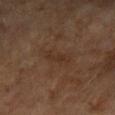The lesion is on the left forearm. A lesion tile, about 15 mm wide, cut from a 3D total-body photograph. The patient is a female roughly 60 years of age. This is a cross-polarized tile. An algorithmic analysis of the crop reported a lesion area of about 3 mm² and a shape-asymmetry score of about 0.35 (0 = symmetric). About 3.5 mm across.